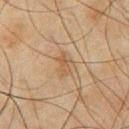Q: Was this lesion biopsied?
A: catalogued during a skin exam; not biopsied
Q: What are the patient's age and sex?
A: male, in their mid-40s
Q: What is the anatomic site?
A: the chest
Q: How was the tile lit?
A: cross-polarized
Q: What is the imaging modality?
A: ~15 mm tile from a whole-body skin photo